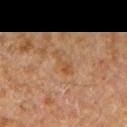The lesion was photographed on a routine skin check and not biopsied; there is no pathology result. Automated image analysis of the tile measured an average lesion color of about L≈46 a*≈18 b*≈33 (CIELAB), about 6 CIELAB-L* units darker than the surrounding skin, and a normalized lesion–skin contrast near 5.5. And it measured a border-irregularity rating of about 5.5/10, internal color variation of about 0.5 on a 0–10 scale, and radial color variation of about 0. It also reported a nevus-likeness score of about 0/100. A male subject about 65 years old. The lesion's longest dimension is about 3.5 mm. Captured under cross-polarized illumination. The lesion is located on the left forearm. A 15 mm crop from a total-body photograph taken for skin-cancer surveillance.